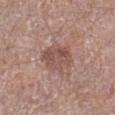Q: Was a biopsy performed?
A: total-body-photography surveillance lesion; no biopsy
Q: Patient demographics?
A: male, in their mid-50s
Q: What is the anatomic site?
A: the right lower leg
Q: How was the tile lit?
A: white-light
Q: What kind of image is this?
A: ~15 mm tile from a whole-body skin photo